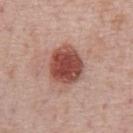The lesion was tiled from a total-body skin photograph and was not biopsied. The tile uses white-light illumination. A 15 mm close-up tile from a total-body photography series done for melanoma screening. Located on the chest. Automated image analysis of the tile measured a footprint of about 15 mm², a shape eccentricity near 0.5, and two-axis asymmetry of about 0.1. It also reported an average lesion color of about L≈48 a*≈26 b*≈26 (CIELAB), roughly 16 lightness units darker than nearby skin, and a normalized lesion–skin contrast near 11. And it measured an automated nevus-likeness rating near 100 out of 100 and lesion-presence confidence of about 100/100. A male subject, about 75 years old.